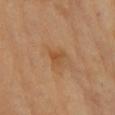Captured during whole-body skin photography for melanoma surveillance; the lesion was not biopsied. Captured under cross-polarized illumination. Approximately 2.5 mm at its widest. A 15 mm close-up extracted from a 3D total-body photography capture. Automated image analysis of the tile measured an area of roughly 3.5 mm², an outline eccentricity of about 0.75 (0 = round, 1 = elongated), and a shape-asymmetry score of about 0.35 (0 = symmetric). It also reported an average lesion color of about L≈51 a*≈21 b*≈38 (CIELAB), roughly 7 lightness units darker than nearby skin, and a lesion-to-skin contrast of about 6 (normalized; higher = more distinct). It also reported border irregularity of about 3.5 on a 0–10 scale, a color-variation rating of about 3/10, and radial color variation of about 1. The software also gave a nevus-likeness score of about 0/100 and lesion-presence confidence of about 100/100. A female patient, aged around 70. From the right upper arm.